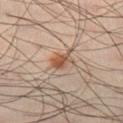notes=catalogued during a skin exam; not biopsied | anatomic site=the leg | lighting=cross-polarized | size=≈3 mm | subject=male, aged 38–42 | image source=total-body-photography crop, ~15 mm field of view.